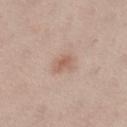follow-up = total-body-photography surveillance lesion; no biopsy | patient = female, aged approximately 25 | automated lesion analysis = a footprint of about 4.5 mm² and an eccentricity of roughly 0.75; an average lesion color of about L≈60 a*≈19 b*≈27 (CIELAB), about 9 CIELAB-L* units darker than the surrounding skin, and a normalized border contrast of about 6; a border-irregularity index near 2.5/10 and a color-variation rating of about 2/10 | image = ~15 mm crop, total-body skin-cancer survey | location = the left lower leg | lighting = white-light | lesion diameter = about 3 mm.